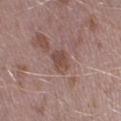Assessment:
Part of a total-body skin-imaging series; this lesion was reviewed on a skin check and was not flagged for biopsy.
Clinical summary:
From the leg. Cropped from a total-body skin-imaging series; the visible field is about 15 mm. A male subject, about 40 years old. This is a white-light tile.